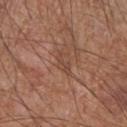Q: Is there a histopathology result?
A: no biopsy performed (imaged during a skin exam)
Q: What kind of image is this?
A: ~15 mm tile from a whole-body skin photo
Q: What is the lesion's diameter?
A: ≈3 mm
Q: Where on the body is the lesion?
A: the right forearm
Q: Automated lesion metrics?
A: a lesion color around L≈45 a*≈21 b*≈28 in CIELAB, a lesion–skin lightness drop of about 6, and a normalized border contrast of about 5; a nevus-likeness score of about 0/100 and lesion-presence confidence of about 75/100
Q: Who is the patient?
A: male, approximately 55 years of age